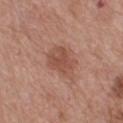Assessment:
Part of a total-body skin-imaging series; this lesion was reviewed on a skin check and was not flagged for biopsy.
Context:
A lesion tile, about 15 mm wide, cut from a 3D total-body photograph. A male patient aged 63–67. This is a white-light tile. Located on the back.